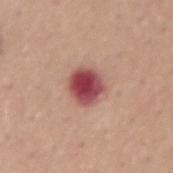<tbp_lesion>
  <biopsy_status>not biopsied; imaged during a skin examination</biopsy_status>
  <lighting>white-light</lighting>
  <lesion_size>
    <long_diameter_mm_approx>3.5</long_diameter_mm_approx>
  </lesion_size>
  <site>lower back</site>
  <image>
    <source>total-body photography crop</source>
    <field_of_view_mm>15</field_of_view_mm>
  </image>
  <patient>
    <sex>female</sex>
    <age_approx>50</age_approx>
  </patient>
</tbp_lesion>